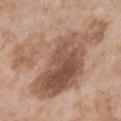biopsy_status: not biopsied; imaged during a skin examination
patient:
  sex: female
  age_approx: 75
lesion_size:
  long_diameter_mm_approx: 13.0
site: chest
lighting: white-light
image:
  source: total-body photography crop
  field_of_view_mm: 15